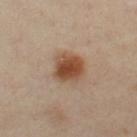workup: total-body-photography surveillance lesion; no biopsy
acquisition: total-body-photography crop, ~15 mm field of view
body site: the right leg
patient: female, roughly 40 years of age